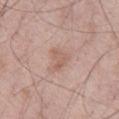Assessment:
This lesion was catalogued during total-body skin photography and was not selected for biopsy.
Image and clinical context:
Approximately 3 mm at its widest. An algorithmic analysis of the crop reported a lesion area of about 4.5 mm² and a symmetry-axis asymmetry near 0.45. The analysis additionally found a border-irregularity rating of about 4.5/10, a within-lesion color-variation index near 2/10, and peripheral color asymmetry of about 0.5. It also reported an automated nevus-likeness rating near 0 out of 100 and lesion-presence confidence of about 100/100. On the right thigh. Captured under white-light illumination. A 15 mm close-up tile from a total-body photography series done for melanoma screening. A male patient, roughly 55 years of age.